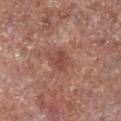Clinical summary:
A male patient aged 53 to 57. The lesion is located on the right lower leg. Captured under white-light illumination. A 15 mm close-up extracted from a 3D total-body photography capture.